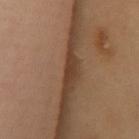Q: Was a biopsy performed?
A: catalogued during a skin exam; not biopsied
Q: What are the patient's age and sex?
A: female, roughly 50 years of age
Q: What is the anatomic site?
A: the chest
Q: What is the imaging modality?
A: ~15 mm tile from a whole-body skin photo
Q: How large is the lesion?
A: ~3 mm (longest diameter)
Q: What lighting was used for the tile?
A: cross-polarized